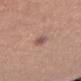Part of a total-body skin-imaging series; this lesion was reviewed on a skin check and was not flagged for biopsy. The lesion is located on the right thigh. Captured under white-light illumination. Automated tile analysis of the lesion measured an area of roughly 3 mm², a shape eccentricity near 0.8, and two-axis asymmetry of about 0.3. A 15 mm close-up tile from a total-body photography series done for melanoma screening. A female subject aged 23 to 27.